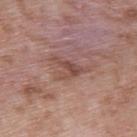Captured during whole-body skin photography for melanoma surveillance; the lesion was not biopsied.
A 15 mm close-up extracted from a 3D total-body photography capture.
The recorded lesion diameter is about 4 mm.
The total-body-photography lesion software estimated a border-irregularity index near 8/10, a within-lesion color-variation index near 2/10, and a peripheral color-asymmetry measure near 0.5.
A male subject, about 50 years old.
From the upper back.
This is a white-light tile.